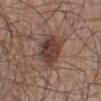The lesion was tiled from a total-body skin photograph and was not biopsied. The total-body-photography lesion software estimated a border-irregularity rating of about 2/10 and radial color variation of about 1.5. Captured under white-light illumination. A male patient, aged 58 to 62. On the left lower leg. Cropped from a whole-body photographic skin survey; the tile spans about 15 mm.